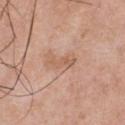Impression: The lesion was photographed on a routine skin check and not biopsied; there is no pathology result. Clinical summary: On the chest. Approximately 3.5 mm at its widest. Captured under white-light illumination. A male subject approximately 55 years of age. A lesion tile, about 15 mm wide, cut from a 3D total-body photograph.